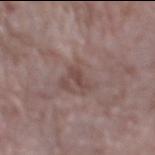notes: imaged on a skin check; not biopsied
subject: female, approximately 70 years of age
site: the right lower leg
acquisition: 15 mm crop, total-body photography
size: ~2.5 mm (longest diameter)
lighting: white-light illumination